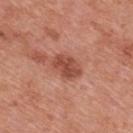workup — no biopsy performed (imaged during a skin exam); location — the mid back; lighting — white-light; size — about 4 mm; acquisition — 15 mm crop, total-body photography; subject — male, roughly 70 years of age.